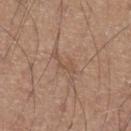<case>
<lesion_size>
  <long_diameter_mm_approx>4.5</long_diameter_mm_approx>
</lesion_size>
<site>leg</site>
<patient>
  <sex>male</sex>
  <age_approx>65</age_approx>
</patient>
<lighting>white-light</lighting>
<image>
  <source>total-body photography crop</source>
  <field_of_view_mm>15</field_of_view_mm>
</image>
</case>